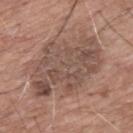Impression: No biopsy was performed on this lesion — it was imaged during a full skin examination and was not determined to be concerning. Image and clinical context: Measured at roughly 8 mm in maximum diameter. The lesion is on the mid back. The lesion-visualizer software estimated a lesion area of about 36 mm², an outline eccentricity of about 0.75 (0 = round, 1 = elongated), and two-axis asymmetry of about 0.3. The software also gave a mean CIELAB color near L≈49 a*≈17 b*≈24 and a lesion-to-skin contrast of about 6.5 (normalized; higher = more distinct). It also reported border irregularity of about 5.5 on a 0–10 scale, internal color variation of about 5 on a 0–10 scale, and a peripheral color-asymmetry measure near 2. The software also gave a classifier nevus-likeness of about 10/100 and lesion-presence confidence of about 100/100. Captured under white-light illumination. A male patient approximately 60 years of age. A region of skin cropped from a whole-body photographic capture, roughly 15 mm wide.